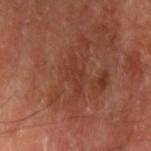Context:
A male subject about 70 years old. Automated tile analysis of the lesion measured a lesion color around L≈36 a*≈26 b*≈29 in CIELAB, roughly 5 lightness units darker than nearby skin, and a normalized lesion–skin contrast near 4.5. The analysis additionally found a within-lesion color-variation index near 0/10 and peripheral color asymmetry of about 0. And it measured a nevus-likeness score of about 0/100 and a lesion-detection confidence of about 100/100. Imaged with cross-polarized lighting. A region of skin cropped from a whole-body photographic capture, roughly 15 mm wide. The recorded lesion diameter is about 3.5 mm. Located on the left upper arm.